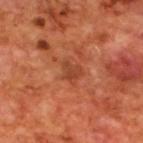{
  "biopsy_status": "not biopsied; imaged during a skin examination",
  "site": "back",
  "lesion_size": {
    "long_diameter_mm_approx": 2.5
  },
  "lighting": "cross-polarized",
  "image": {
    "source": "total-body photography crop",
    "field_of_view_mm": 15
  },
  "automated_metrics": {
    "eccentricity": 0.6,
    "shape_asymmetry": 0.4,
    "cielab_L": 43,
    "cielab_a": 29,
    "cielab_b": 35,
    "vs_skin_darker_L": 8.0,
    "vs_skin_contrast_norm": 6.0,
    "border_irregularity_0_10": 3.5,
    "color_variation_0_10": 2.0,
    "peripheral_color_asymmetry": 0.5
  },
  "patient": {
    "sex": "male",
    "age_approx": 70
  }
}